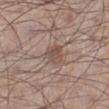Impression: Part of a total-body skin-imaging series; this lesion was reviewed on a skin check and was not flagged for biopsy. Image and clinical context: This image is a 15 mm lesion crop taken from a total-body photograph. The patient is a male approximately 55 years of age. The lesion-visualizer software estimated a footprint of about 6 mm², an outline eccentricity of about 0.55 (0 = round, 1 = elongated), and a shape-asymmetry score of about 0.25 (0 = symmetric). The analysis additionally found an average lesion color of about L≈50 a*≈17 b*≈24 (CIELAB) and roughly 8 lightness units darker than nearby skin. It also reported a border-irregularity rating of about 2.5/10 and a within-lesion color-variation index near 3/10. And it measured a detector confidence of about 100 out of 100 that the crop contains a lesion. The tile uses white-light illumination. Longest diameter approximately 3 mm. The lesion is located on the left lower leg.